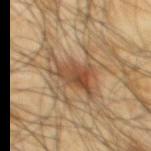workup: catalogued during a skin exam; not biopsied
size: ≈5.5 mm
anatomic site: the upper back
patient: male, in their mid- to late 60s
automated metrics: a lesion area of about 16 mm², an outline eccentricity of about 0.75 (0 = round, 1 = elongated), and two-axis asymmetry of about 0.4
imaging modality: ~15 mm crop, total-body skin-cancer survey
tile lighting: cross-polarized illumination